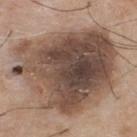Case summary:
* notes · no biopsy performed (imaged during a skin exam)
* lighting · white-light
* anatomic site · the back
* subject · male, aged 73 to 77
* lesion size · ~12 mm (longest diameter)
* image · 15 mm crop, total-body photography